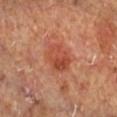Assessment:
Captured during whole-body skin photography for melanoma surveillance; the lesion was not biopsied.
Context:
A close-up tile cropped from a whole-body skin photograph, about 15 mm across. Imaged with cross-polarized lighting. The lesion-visualizer software estimated an eccentricity of roughly 0.6. The lesion is located on the right lower leg. A male subject, aged 63 to 67.